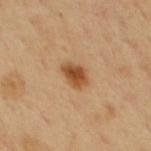biopsy_status: not biopsied; imaged during a skin examination
site: chest
patient:
  sex: male
  age_approx: 50
image:
  source: total-body photography crop
  field_of_view_mm: 15